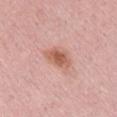notes = no biopsy performed (imaged during a skin exam) | lighting = white-light illumination | image = ~15 mm crop, total-body skin-cancer survey | body site = the chest | patient = female, aged 68–72 | lesion size = ~3.5 mm (longest diameter).